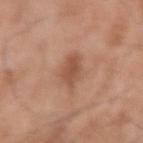Imaged during a routine full-body skin examination; the lesion was not biopsied and no histopathology is available.
Captured under white-light illumination.
The lesion is located on the right upper arm.
A lesion tile, about 15 mm wide, cut from a 3D total-body photograph.
The patient is a male roughly 55 years of age.